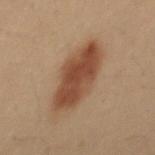Impression: Captured during whole-body skin photography for melanoma surveillance; the lesion was not biopsied. Background: From the mid back. The tile uses cross-polarized illumination. A male subject about 30 years old. The recorded lesion diameter is about 7 mm. Automated tile analysis of the lesion measured an area of roughly 22 mm², an outline eccentricity of about 0.85 (0 = round, 1 = elongated), and two-axis asymmetry of about 0.15. It also reported border irregularity of about 2 on a 0–10 scale, a within-lesion color-variation index near 4/10, and a peripheral color-asymmetry measure near 1.5. The software also gave an automated nevus-likeness rating near 100 out of 100 and a lesion-detection confidence of about 100/100. A region of skin cropped from a whole-body photographic capture, roughly 15 mm wide.